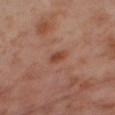follow-up: total-body-photography surveillance lesion; no biopsy
acquisition: 15 mm crop, total-body photography
patient: female, approximately 55 years of age
site: the right thigh